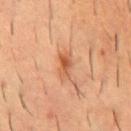Impression: Captured during whole-body skin photography for melanoma surveillance; the lesion was not biopsied. Context: A male subject aged around 55. The lesion's longest dimension is about 3.5 mm. Cropped from a total-body skin-imaging series; the visible field is about 15 mm. The tile uses cross-polarized illumination. The lesion is located on the mid back.